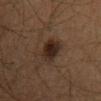<lesion>
  <site>mid back</site>
  <image>
    <source>total-body photography crop</source>
    <field_of_view_mm>15</field_of_view_mm>
  </image>
  <patient>
    <sex>male</sex>
    <age_approx>65</age_approx>
  </patient>
</lesion>